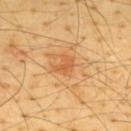biopsy status = total-body-photography surveillance lesion; no biopsy | TBP lesion metrics = a mean CIELAB color near L≈61 a*≈27 b*≈45 and a normalized lesion–skin contrast near 5.5; an automated nevus-likeness rating near 25 out of 100 and a detector confidence of about 100 out of 100 that the crop contains a lesion | image source = 15 mm crop, total-body photography | site = the back | diameter = ~2.5 mm (longest diameter) | patient = male, approximately 65 years of age.